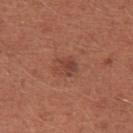Part of a total-body skin-imaging series; this lesion was reviewed on a skin check and was not flagged for biopsy.